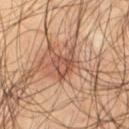notes=imaged on a skin check; not biopsied | patient=male, aged around 55 | anatomic site=the left thigh | imaging modality=15 mm crop, total-body photography.